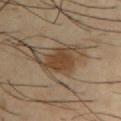| feature | finding |
|---|---|
| notes | total-body-photography surveillance lesion; no biopsy |